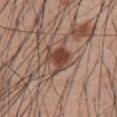| key | value |
|---|---|
| workup | imaged on a skin check; not biopsied |
| imaging modality | ~15 mm crop, total-body skin-cancer survey |
| size | ~4 mm (longest diameter) |
| site | the abdomen |
| tile lighting | white-light |
| patient | male, in their mid- to late 50s |